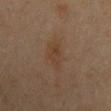<record>
  <biopsy_status>not biopsied; imaged during a skin examination</biopsy_status>
</record>